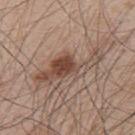| key | value |
|---|---|
| follow-up | total-body-photography surveillance lesion; no biopsy |
| image | ~15 mm crop, total-body skin-cancer survey |
| site | the upper back |
| patient | male, about 50 years old |